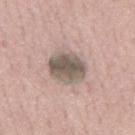Part of a total-body skin-imaging series; this lesion was reviewed on a skin check and was not flagged for biopsy. An algorithmic analysis of the crop reported a mean CIELAB color near L≈55 a*≈9 b*≈22, a lesion–skin lightness drop of about 14, and a normalized border contrast of about 9. Imaged with white-light lighting. A male subject, aged around 65. Measured at roughly 4 mm in maximum diameter. Cropped from a total-body skin-imaging series; the visible field is about 15 mm. The lesion is on the mid back.